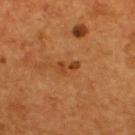Recorded during total-body skin imaging; not selected for excision or biopsy. The tile uses cross-polarized illumination. A close-up tile cropped from a whole-body skin photograph, about 15 mm across. The lesion's longest dimension is about 2.5 mm. An algorithmic analysis of the crop reported a footprint of about 3 mm², an outline eccentricity of about 0.9 (0 = round, 1 = elongated), and a symmetry-axis asymmetry near 0.5. It also reported an average lesion color of about L≈39 a*≈25 b*≈37 (CIELAB) and a normalized lesion–skin contrast near 7. The software also gave a border-irregularity rating of about 5.5/10 and a within-lesion color-variation index near 0/10. A male subject, aged around 55. From the upper back.